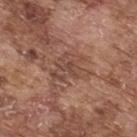Impression:
No biopsy was performed on this lesion — it was imaged during a full skin examination and was not determined to be concerning.
Image and clinical context:
Approximately 4.5 mm at its widest. A male patient roughly 75 years of age. Captured under white-light illumination. On the upper back. A 15 mm close-up tile from a total-body photography series done for melanoma screening.